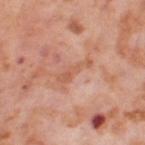This lesion was catalogued during total-body skin photography and was not selected for biopsy.
A female patient, aged around 55.
Captured under cross-polarized illumination.
Cropped from a whole-body photographic skin survey; the tile spans about 15 mm.
Measured at roughly 4 mm in maximum diameter.
From the leg.